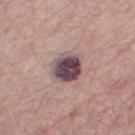Q: Is there a histopathology result?
A: no biopsy performed (imaged during a skin exam)
Q: What is the lesion's diameter?
A: ~3.5 mm (longest diameter)
Q: Where on the body is the lesion?
A: the right thigh
Q: How was the tile lit?
A: white-light illumination
Q: What is the imaging modality?
A: 15 mm crop, total-body photography
Q: What are the patient's age and sex?
A: female, aged 63 to 67